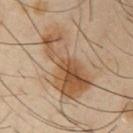Cropped from a whole-body photographic skin survey; the tile spans about 15 mm.
From the right upper arm.
The patient is a male aged around 40.